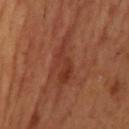No biopsy was performed on this lesion — it was imaged during a full skin examination and was not determined to be concerning.
On the head or neck.
Approximately 6.5 mm at its widest.
The patient is a male approximately 55 years of age.
A 15 mm crop from a total-body photograph taken for skin-cancer surveillance.
Captured under cross-polarized illumination.
Automated tile analysis of the lesion measured an average lesion color of about L≈35 a*≈26 b*≈30 (CIELAB) and a lesion–skin lightness drop of about 7. The software also gave border irregularity of about 6.5 on a 0–10 scale, a within-lesion color-variation index near 3.5/10, and radial color variation of about 1.